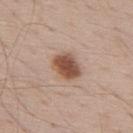Part of a total-body skin-imaging series; this lesion was reviewed on a skin check and was not flagged for biopsy. An algorithmic analysis of the crop reported a mean CIELAB color near L≈51 a*≈20 b*≈29, about 15 CIELAB-L* units darker than the surrounding skin, and a normalized lesion–skin contrast near 10.5. And it measured a border-irregularity index near 1.5/10, a within-lesion color-variation index near 3.5/10, and a peripheral color-asymmetry measure near 1. The lesion is on the upper back. The tile uses white-light illumination. The recorded lesion diameter is about 3.5 mm. A 15 mm crop from a total-body photograph taken for skin-cancer surveillance. A male subject, aged 68–72.